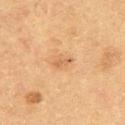This lesion was catalogued during total-body skin photography and was not selected for biopsy. Imaged with cross-polarized lighting. The lesion-visualizer software estimated a mean CIELAB color near L≈55 a*≈20 b*≈36, a lesion–skin lightness drop of about 8, and a normalized lesion–skin contrast near 5.5. The analysis additionally found a border-irregularity index near 3/10, internal color variation of about 0 on a 0–10 scale, and a peripheral color-asymmetry measure near 0. It also reported a classifier nevus-likeness of about 0/100. A 15 mm close-up tile from a total-body photography series done for melanoma screening. About 2.5 mm across. A male patient roughly 60 years of age.